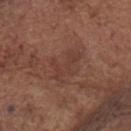The lesion was photographed on a routine skin check and not biopsied; there is no pathology result.
The lesion is located on the head or neck.
A region of skin cropped from a whole-body photographic capture, roughly 15 mm wide.
A male patient aged approximately 80.
Approximately 4.5 mm at its widest.
This is a white-light tile.